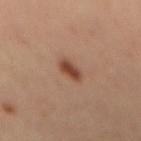biopsy status: total-body-photography surveillance lesion; no biopsy
automated metrics: an area of roughly 4.5 mm² and a shape eccentricity near 0.85; an average lesion color of about L≈46 a*≈23 b*≈31 (CIELAB) and a lesion-to-skin contrast of about 9.5 (normalized; higher = more distinct); a border-irregularity index near 2.5/10 and internal color variation of about 3.5 on a 0–10 scale; a classifier nevus-likeness of about 95/100
site: the mid back
size: ~3 mm (longest diameter)
illumination: cross-polarized
image source: ~15 mm crop, total-body skin-cancer survey
subject: female, in their mid-30s